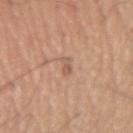Part of a total-body skin-imaging series; this lesion was reviewed on a skin check and was not flagged for biopsy. The subject is a male about 60 years old. The lesion is on the right upper arm. The recorded lesion diameter is about 2.5 mm. This is a white-light tile. A close-up tile cropped from a whole-body skin photograph, about 15 mm across.